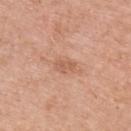| feature | finding |
|---|---|
| workup | total-body-photography surveillance lesion; no biopsy |
| imaging modality | ~15 mm tile from a whole-body skin photo |
| body site | the left upper arm |
| patient | female, aged around 60 |
| lesion size | ≈2.5 mm |
| illumination | white-light |